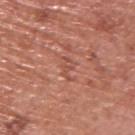Recorded during total-body skin imaging; not selected for excision or biopsy. A male subject roughly 75 years of age. A region of skin cropped from a whole-body photographic capture, roughly 15 mm wide. From the upper back. About 3 mm across. The tile uses white-light illumination.